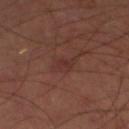follow-up: no biopsy performed (imaged during a skin exam) | size: ~3 mm (longest diameter) | automated lesion analysis: an area of roughly 4 mm² and a shape eccentricity near 0.8; a peripheral color-asymmetry measure near 0.5 | patient: male, in their mid-60s | image: total-body-photography crop, ~15 mm field of view | body site: the left lower leg.